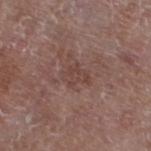Q: Was a biopsy performed?
A: no biopsy performed (imaged during a skin exam)
Q: Lesion location?
A: the left lower leg
Q: How large is the lesion?
A: ~3 mm (longest diameter)
Q: What kind of image is this?
A: 15 mm crop, total-body photography
Q: How was the tile lit?
A: white-light illumination
Q: Who is the patient?
A: male, aged 78–82